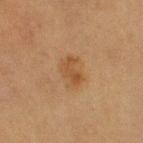Case summary:
• notes: no biopsy performed (imaged during a skin exam)
• image source: ~15 mm crop, total-body skin-cancer survey
• site: the right upper arm
• subject: female, aged approximately 55